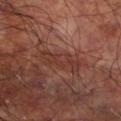No biopsy was performed on this lesion — it was imaged during a full skin examination and was not determined to be concerning.
A male patient, aged 58 to 62.
The lesion is located on the right lower leg.
A 15 mm close-up tile from a total-body photography series done for melanoma screening.